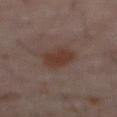<lesion>
<biopsy_status>not biopsied; imaged during a skin examination</biopsy_status>
<image>
  <source>total-body photography crop</source>
  <field_of_view_mm>15</field_of_view_mm>
</image>
<lesion_size>
  <long_diameter_mm_approx>4.0</long_diameter_mm_approx>
</lesion_size>
<lighting>cross-polarized</lighting>
<automated_metrics>
  <eccentricity>0.7</eccentricity>
  <shape_asymmetry>0.25</shape_asymmetry>
  <vs_skin_contrast_norm>8.5</vs_skin_contrast_norm>
  <nevus_likeness_0_100>100</nevus_likeness_0_100>
  <lesion_detection_confidence_0_100>100</lesion_detection_confidence_0_100>
</automated_metrics>
<patient>
  <sex>male</sex>
  <age_approx>60</age_approx>
</patient>
<site>abdomen</site>
</lesion>